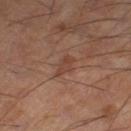Clinical impression: The lesion was tiled from a total-body skin photograph and was not biopsied. Acquisition and patient details: Automated image analysis of the tile measured an outline eccentricity of about 0.9 (0 = round, 1 = elongated). It also reported an automated nevus-likeness rating near 5 out of 100 and a lesion-detection confidence of about 100/100. The lesion is located on the leg. This is a cross-polarized tile. Cropped from a whole-body photographic skin survey; the tile spans about 15 mm. A male patient, approximately 55 years of age.